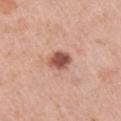Notes:
• workup — imaged on a skin check; not biopsied
• patient — female, approximately 40 years of age
• TBP lesion metrics — a lesion color around L≈53 a*≈24 b*≈28 in CIELAB, a lesion–skin lightness drop of about 16, and a normalized lesion–skin contrast near 10.5; peripheral color asymmetry of about 1.5; an automated nevus-likeness rating near 80 out of 100
• acquisition — total-body-photography crop, ~15 mm field of view
• location — the right upper arm
• lighting — white-light illumination
• lesion size — about 2.5 mm